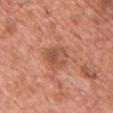Recorded during total-body skin imaging; not selected for excision or biopsy. A female patient, roughly 50 years of age. A close-up tile cropped from a whole-body skin photograph, about 15 mm across. Located on the chest. The tile uses white-light illumination. The recorded lesion diameter is about 3 mm.